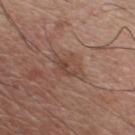notes: no biopsy performed (imaged during a skin exam); diameter: ~4 mm (longest diameter); image: 15 mm crop, total-body photography; subject: male, aged approximately 75; location: the chest; tile lighting: white-light illumination.